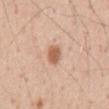biopsy_status: not biopsied; imaged during a skin examination
patient:
  sex: male
  age_approx: 55
image:
  source: total-body photography crop
  field_of_view_mm: 15
site: abdomen
lesion_size:
  long_diameter_mm_approx: 2.5
lighting: white-light